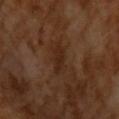Findings:
- workup · total-body-photography surveillance lesion; no biopsy